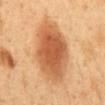Notes:
- notes — imaged on a skin check; not biopsied
- subject — male, aged 48 to 52
- diameter — about 8 mm
- site — the abdomen
- acquisition — ~15 mm crop, total-body skin-cancer survey